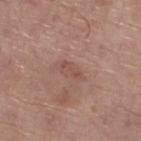The lesion was photographed on a routine skin check and not biopsied; there is no pathology result. On the leg. A male patient in their 70s. Cropped from a total-body skin-imaging series; the visible field is about 15 mm.